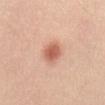biopsy_status: not biopsied; imaged during a skin examination
site: abdomen
lesion_size:
  long_diameter_mm_approx: 3.0
image:
  source: total-body photography crop
  field_of_view_mm: 15
patient:
  sex: female
  age_approx: 50